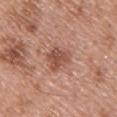notes: imaged on a skin check; not biopsied
imaging modality: ~15 mm crop, total-body skin-cancer survey
subject: male, roughly 50 years of age
diameter: ~3.5 mm (longest diameter)
image-analysis metrics: a nevus-likeness score of about 45/100 and a detector confidence of about 100 out of 100 that the crop contains a lesion
anatomic site: the upper back